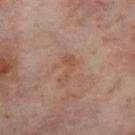Clinical impression: Imaged during a routine full-body skin examination; the lesion was not biopsied and no histopathology is available. Background: Measured at roughly 3.5 mm in maximum diameter. The lesion is located on the leg. An algorithmic analysis of the crop reported a footprint of about 4.5 mm² and an outline eccentricity of about 0.9 (0 = round, 1 = elongated). A 15 mm crop from a total-body photograph taken for skin-cancer surveillance. This is a cross-polarized tile. The subject is a female aged 53 to 57.